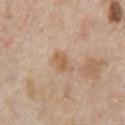biopsy status: no biopsy performed (imaged during a skin exam)
size: about 3 mm
automated metrics: a lesion area of about 4.5 mm², a shape eccentricity near 0.75, and two-axis asymmetry of about 0.3; a border-irregularity index near 3/10, internal color variation of about 2 on a 0–10 scale, and radial color variation of about 0.5
acquisition: total-body-photography crop, ~15 mm field of view
patient: male, aged 63–67
body site: the chest